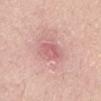biopsy_status: not biopsied; imaged during a skin examination
lesion_size:
  long_diameter_mm_approx: 2.5
site: back
image:
  source: total-body photography crop
  field_of_view_mm: 15
lighting: white-light
patient:
  sex: male
  age_approx: 50
automated_metrics:
  cielab_L: 60
  cielab_a: 29
  cielab_b: 22
  border_irregularity_0_10: 2.0
  color_variation_0_10: 3.5
  lesion_detection_confidence_0_100: 100